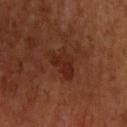Q: Was this lesion biopsied?
A: total-body-photography surveillance lesion; no biopsy
Q: What is the lesion's diameter?
A: ~4 mm (longest diameter)
Q: What kind of image is this?
A: 15 mm crop, total-body photography
Q: How was the tile lit?
A: cross-polarized illumination
Q: Automated lesion metrics?
A: an eccentricity of roughly 0.75 and a symmetry-axis asymmetry near 0.45; a classifier nevus-likeness of about 0/100 and a lesion-detection confidence of about 100/100
Q: Lesion location?
A: the left upper arm
Q: Patient demographics?
A: female, aged 63 to 67